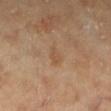No biopsy was performed on this lesion — it was imaged during a full skin examination and was not determined to be concerning.
The lesion is located on the left lower leg.
Cropped from a total-body skin-imaging series; the visible field is about 15 mm.
The patient is a female in their 60s.